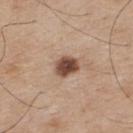<tbp_lesion>
<biopsy_status>not biopsied; imaged during a skin examination</biopsy_status>
<lighting>white-light</lighting>
<image>
  <source>total-body photography crop</source>
  <field_of_view_mm>15</field_of_view_mm>
</image>
<patient>
  <sex>male</sex>
  <age_approx>75</age_approx>
</patient>
<lesion_size>
  <long_diameter_mm_approx>3.0</long_diameter_mm_approx>
</lesion_size>
<site>back</site>
</tbp_lesion>